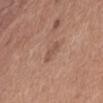  biopsy_status: not biopsied; imaged during a skin examination